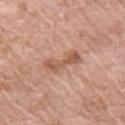<case>
  <biopsy_status>not biopsied; imaged during a skin examination</biopsy_status>
  <lesion_size>
    <long_diameter_mm_approx>4.0</long_diameter_mm_approx>
  </lesion_size>
  <patient>
    <sex>female</sex>
    <age_approx>75</age_approx>
  </patient>
  <image>
    <source>total-body photography crop</source>
    <field_of_view_mm>15</field_of_view_mm>
  </image>
  <lighting>white-light</lighting>
  <site>right upper arm</site>
  <automated_metrics>
    <area_mm2_approx>6.5</area_mm2_approx>
    <eccentricity>0.9</eccentricity>
    <shape_asymmetry>0.35</shape_asymmetry>
  </automated_metrics>
</case>